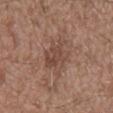Recorded during total-body skin imaging; not selected for excision or biopsy.
Located on the mid back.
About 5.5 mm across.
A male subject roughly 75 years of age.
Cropped from a whole-body photographic skin survey; the tile spans about 15 mm.